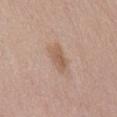workup: catalogued during a skin exam; not biopsied